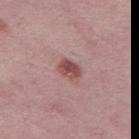Impression:
Recorded during total-body skin imaging; not selected for excision or biopsy.
Image and clinical context:
A 15 mm crop from a total-body photograph taken for skin-cancer surveillance. Measured at roughly 3 mm in maximum diameter. Located on the left thigh. An algorithmic analysis of the crop reported a lesion color around L≈49 a*≈24 b*≈21 in CIELAB, about 12 CIELAB-L* units darker than the surrounding skin, and a normalized lesion–skin contrast near 9. And it measured border irregularity of about 1.5 on a 0–10 scale and a within-lesion color-variation index near 2.5/10. The patient is a female in their mid-40s. Captured under white-light illumination.